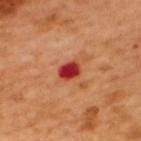Assessment: Captured during whole-body skin photography for melanoma surveillance; the lesion was not biopsied. Background: The lesion is located on the upper back. The subject is a male aged approximately 50. Cropped from a whole-body photographic skin survey; the tile spans about 15 mm.